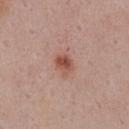| field | value |
|---|---|
| biopsy status | total-body-photography surveillance lesion; no biopsy |
| tile lighting | white-light illumination |
| TBP lesion metrics | a border-irregularity rating of about 2/10 and a peripheral color-asymmetry measure near 1.5; a classifier nevus-likeness of about 90/100 and a lesion-detection confidence of about 100/100 |
| acquisition | ~15 mm tile from a whole-body skin photo |
| patient | female, roughly 55 years of age |
| lesion size | ~2.5 mm (longest diameter) |
| site | the front of the torso |